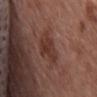workup: imaged on a skin check; not biopsied
size: ≈4 mm
imaging modality: ~15 mm crop, total-body skin-cancer survey
subject: female, aged around 65
automated metrics: a mean CIELAB color near L≈36 a*≈21 b*≈24, roughly 7 lightness units darker than nearby skin, and a lesion-to-skin contrast of about 7 (normalized; higher = more distinct); an automated nevus-likeness rating near 10 out of 100
anatomic site: the upper back